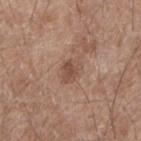No biopsy was performed on this lesion — it was imaged during a full skin examination and was not determined to be concerning. A roughly 15 mm field-of-view crop from a total-body skin photograph. A male subject approximately 60 years of age. Automated tile analysis of the lesion measured a lesion area of about 4.5 mm², an eccentricity of roughly 0.65, and a symmetry-axis asymmetry near 0.25. The software also gave a lesion color around L≈49 a*≈20 b*≈27 in CIELAB, roughly 9 lightness units darker than nearby skin, and a lesion-to-skin contrast of about 6.5 (normalized; higher = more distinct). The software also gave a nevus-likeness score of about 5/100 and a lesion-detection confidence of about 100/100. Approximately 2.5 mm at its widest. The lesion is located on the right forearm. Captured under white-light illumination.